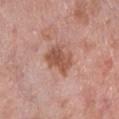  biopsy_status: not biopsied; imaged during a skin examination
  lesion_size:
    long_diameter_mm_approx: 4.0
  patient:
    sex: female
    age_approx: 50
  automated_metrics:
    area_mm2_approx: 9.0
    eccentricity: 0.7
    shape_asymmetry: 0.25
    border_irregularity_0_10: 3.0
    color_variation_0_10: 3.0
    nevus_likeness_0_100: 10
    lesion_detection_confidence_0_100: 100
  image:
    source: total-body photography crop
    field_of_view_mm: 15
  site: left lower leg
  lighting: white-light